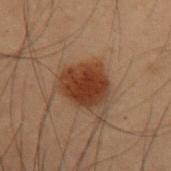The lesion was photographed on a routine skin check and not biopsied; there is no pathology result.
On the left upper arm.
A male subject roughly 55 years of age.
A close-up tile cropped from a whole-body skin photograph, about 15 mm across.